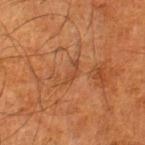follow-up = catalogued during a skin exam; not biopsied | patient = male, aged 63–67 | location = the leg | image-analysis metrics = a mean CIELAB color near L≈37 a*≈21 b*≈31, about 5 CIELAB-L* units darker than the surrounding skin, and a normalized lesion–skin contrast near 5; a border-irregularity index near 5.5/10 and internal color variation of about 0 on a 0–10 scale | diameter = ≈3 mm | tile lighting = cross-polarized illumination | image source = 15 mm crop, total-body photography.